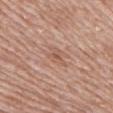Clinical impression: Captured during whole-body skin photography for melanoma surveillance; the lesion was not biopsied. Clinical summary: Automated image analysis of the tile measured a shape eccentricity near 0.75 and a shape-asymmetry score of about 0.55 (0 = symmetric). The software also gave a nevus-likeness score of about 0/100 and a lesion-detection confidence of about 100/100. The tile uses white-light illumination. Longest diameter approximately 3 mm. A region of skin cropped from a whole-body photographic capture, roughly 15 mm wide. A male subject approximately 70 years of age. The lesion is on the right upper arm.